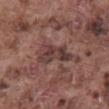| feature | finding |
|---|---|
| follow-up | total-body-photography surveillance lesion; no biopsy |
| lesion diameter | ~5 mm (longest diameter) |
| image source | ~15 mm tile from a whole-body skin photo |
| anatomic site | the abdomen |
| subject | male, aged approximately 75 |
| automated lesion analysis | a footprint of about 10 mm² and a shape-asymmetry score of about 0.4 (0 = symmetric); a border-irregularity index near 5/10, a within-lesion color-variation index near 5/10, and a peripheral color-asymmetry measure near 2 |